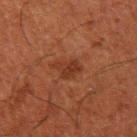Imaged during a routine full-body skin examination; the lesion was not biopsied and no histopathology is available. The lesion-visualizer software estimated a border-irregularity rating of about 3.5/10, a within-lesion color-variation index near 2/10, and radial color variation of about 0.5. And it measured a nevus-likeness score of about 10/100. This is a cross-polarized tile. A lesion tile, about 15 mm wide, cut from a 3D total-body photograph. The patient is a male aged around 50. Approximately 3 mm at its widest. On the left upper arm.